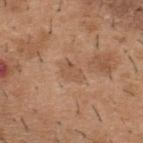workup: no biopsy performed (imaged during a skin exam) | imaging modality: total-body-photography crop, ~15 mm field of view | site: the upper back | lighting: white-light illumination | automated metrics: a mean CIELAB color near L≈53 a*≈19 b*≈32, roughly 7 lightness units darker than nearby skin, and a lesion-to-skin contrast of about 5 (normalized; higher = more distinct) | diameter: ≈3 mm | subject: male, aged approximately 40.